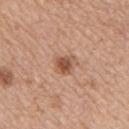notes = imaged on a skin check; not biopsied
site = the chest
image = total-body-photography crop, ~15 mm field of view
illumination = white-light
subject = female, in their mid-40s